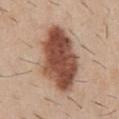Assessment:
Part of a total-body skin-imaging series; this lesion was reviewed on a skin check and was not flagged for biopsy.
Context:
About 8.5 mm across. A male subject roughly 30 years of age. The lesion-visualizer software estimated a mean CIELAB color near L≈49 a*≈22 b*≈29, a lesion–skin lightness drop of about 18, and a normalized border contrast of about 12.5. It also reported a border-irregularity index near 2/10 and a within-lesion color-variation index near 6/10. Cropped from a whole-body photographic skin survey; the tile spans about 15 mm. The lesion is located on the front of the torso. Captured under white-light illumination.